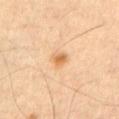The lesion was photographed on a routine skin check and not biopsied; there is no pathology result.
On the front of the torso.
The subject is a male aged approximately 55.
This image is a 15 mm lesion crop taken from a total-body photograph.
Measured at roughly 2 mm in maximum diameter.